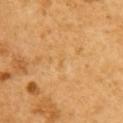{
  "biopsy_status": "not biopsied; imaged during a skin examination",
  "lighting": "cross-polarized",
  "site": "upper back",
  "patient": {
    "sex": "female",
    "age_approx": 55
  },
  "lesion_size": {
    "long_diameter_mm_approx": 1.5
  },
  "image": {
    "source": "total-body photography crop",
    "field_of_view_mm": 15
  }
}